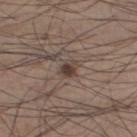The lesion was tiled from a total-body skin photograph and was not biopsied.
Captured under white-light illumination.
The lesion's longest dimension is about 2.5 mm.
The lesion is located on the leg.
A 15 mm crop from a total-body photograph taken for skin-cancer surveillance.
Automated image analysis of the tile measured an area of roughly 4 mm² and an outline eccentricity of about 0.65 (0 = round, 1 = elongated). The analysis additionally found a lesion–skin lightness drop of about 10 and a normalized lesion–skin contrast near 8. The analysis additionally found border irregularity of about 1.5 on a 0–10 scale, internal color variation of about 5 on a 0–10 scale, and peripheral color asymmetry of about 2. The software also gave a classifier nevus-likeness of about 95/100.
The patient is a male aged approximately 35.